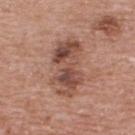body site — the back | diameter — ~7.5 mm (longest diameter) | subject — male, aged 68–72 | illumination — white-light illumination | acquisition — ~15 mm tile from a whole-body skin photo.